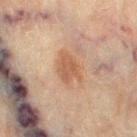{
  "biopsy_status": "not biopsied; imaged during a skin examination",
  "image": {
    "source": "total-body photography crop",
    "field_of_view_mm": 15
  },
  "site": "leg",
  "automated_metrics": {
    "area_mm2_approx": 7.5,
    "eccentricity": 0.65,
    "shape_asymmetry": 0.3,
    "vs_skin_darker_L": 8.0,
    "vs_skin_contrast_norm": 6.5
  },
  "patient": {
    "sex": "female",
    "age_approx": 80
  },
  "lesion_size": {
    "long_diameter_mm_approx": 4.0
  },
  "lighting": "cross-polarized"
}